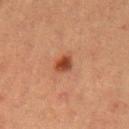Recorded during total-body skin imaging; not selected for excision or biopsy.
This is a cross-polarized tile.
The lesion is on the left upper arm.
A 15 mm crop from a total-body photograph taken for skin-cancer surveillance.
A female subject, roughly 55 years of age.